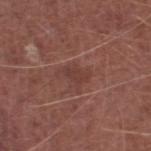Findings:
• biopsy status · imaged on a skin check; not biopsied
• image-analysis metrics · an automated nevus-likeness rating near 0 out of 100 and lesion-presence confidence of about 100/100
• tile lighting · white-light illumination
• subject · male, in their mid-70s
• image source · 15 mm crop, total-body photography
• body site · the right lower leg